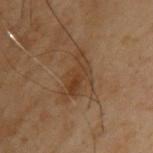Context:
Imaged with cross-polarized lighting. Measured at roughly 5 mm in maximum diameter. A male subject in their mid-50s. The total-body-photography lesion software estimated a lesion area of about 8.5 mm², an eccentricity of roughly 0.9, and two-axis asymmetry of about 0.45. The software also gave a lesion color around L≈31 a*≈15 b*≈26 in CIELAB, a lesion–skin lightness drop of about 6, and a normalized lesion–skin contrast near 6.5. On the upper back. A lesion tile, about 15 mm wide, cut from a 3D total-body photograph.